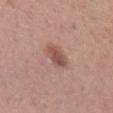Assessment: The lesion was photographed on a routine skin check and not biopsied; there is no pathology result. Context: On the left forearm. A roughly 15 mm field-of-view crop from a total-body skin photograph. An algorithmic analysis of the crop reported an automated nevus-likeness rating near 65 out of 100. Imaged with white-light lighting. Approximately 3 mm at its widest. The patient is a female aged approximately 30.